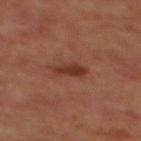| field | value |
|---|---|
| follow-up | catalogued during a skin exam; not biopsied |
| anatomic site | the mid back |
| size | ~3 mm (longest diameter) |
| subject | male, aged approximately 70 |
| imaging modality | ~15 mm crop, total-body skin-cancer survey |
| tile lighting | cross-polarized illumination |